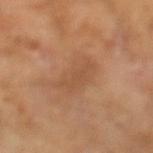This lesion was catalogued during total-body skin photography and was not selected for biopsy. Located on the left lower leg. Automated image analysis of the tile measured a footprint of about 9.5 mm², an outline eccentricity of about 0.9 (0 = round, 1 = elongated), and a symmetry-axis asymmetry near 0.35. It also reported about 6 CIELAB-L* units darker than the surrounding skin and a lesion-to-skin contrast of about 4.5 (normalized; higher = more distinct). Measured at roughly 5.5 mm in maximum diameter. This image is a 15 mm lesion crop taken from a total-body photograph. This is a cross-polarized tile. The subject is a male about 65 years old.